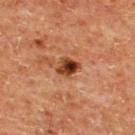Clinical impression:
Recorded during total-body skin imaging; not selected for excision or biopsy.